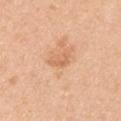workup = imaged on a skin check; not biopsied | body site = the right upper arm | image source = ~15 mm crop, total-body skin-cancer survey | lighting = white-light illumination | lesion size = ~3 mm (longest diameter) | patient = male, aged 48–52.